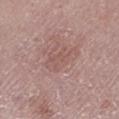workup = imaged on a skin check; not biopsied
image = total-body-photography crop, ~15 mm field of view
subject = female, about 65 years old
lesion diameter = about 4.5 mm
lighting = white-light
anatomic site = the left lower leg